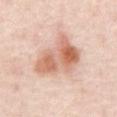Clinical impression: Part of a total-body skin-imaging series; this lesion was reviewed on a skin check and was not flagged for biopsy. Image and clinical context: Cropped from a whole-body photographic skin survey; the tile spans about 15 mm. A patient aged 53 to 57. The lesion's longest dimension is about 6 mm. From the abdomen. The tile uses cross-polarized illumination. The lesion-visualizer software estimated a footprint of about 19 mm², an eccentricity of roughly 0.75, and a shape-asymmetry score of about 0.35 (0 = symmetric). And it measured a lesion–skin lightness drop of about 13 and a normalized lesion–skin contrast near 8.5.